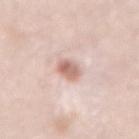Clinical impression: Imaged during a routine full-body skin examination; the lesion was not biopsied and no histopathology is available. Background: A 15 mm close-up extracted from a 3D total-body photography capture. The subject is a female aged 48 to 52. On the mid back.